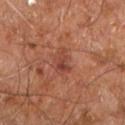Notes:
* lesion size — ~3 mm (longest diameter)
* location — the right leg
* patient — male, aged around 60
* acquisition — 15 mm crop, total-body photography
* tile lighting — cross-polarized